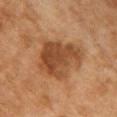subject: female, in their 60s | location: the chest | diameter: ~5.5 mm (longest diameter) | TBP lesion metrics: a footprint of about 21 mm²; an average lesion color of about L≈40 a*≈20 b*≈32 (CIELAB), roughly 10 lightness units darker than nearby skin, and a normalized lesion–skin contrast near 8.5; border irregularity of about 3.5 on a 0–10 scale and a peripheral color-asymmetry measure near 1.5; a nevus-likeness score of about 20/100 and lesion-presence confidence of about 100/100 | acquisition: ~15 mm crop, total-body skin-cancer survey | lighting: cross-polarized illumination.